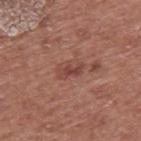Background:
A lesion tile, about 15 mm wide, cut from a 3D total-body photograph. This is a white-light tile. The total-body-photography lesion software estimated a lesion–skin lightness drop of about 8 and a lesion-to-skin contrast of about 6.5 (normalized; higher = more distinct). The analysis additionally found internal color variation of about 2 on a 0–10 scale and radial color variation of about 0.5. About 3 mm across. On the upper back. A male patient in their mid-70s.